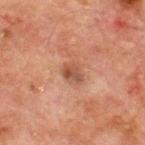Part of a total-body skin-imaging series; this lesion was reviewed on a skin check and was not flagged for biopsy.
Cropped from a total-body skin-imaging series; the visible field is about 15 mm.
A male subject, aged 63–67.
The lesion is on the front of the torso.